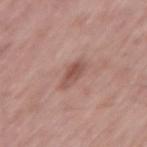follow-up = total-body-photography surveillance lesion; no biopsy | tile lighting = white-light | diameter = ≈3 mm | site = the left thigh | patient = male, in their 70s | acquisition = ~15 mm tile from a whole-body skin photo.